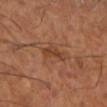• biopsy status · imaged on a skin check; not biopsied
• site · the leg
• image · 15 mm crop, total-body photography
• tile lighting · cross-polarized illumination
• size · ≈3.5 mm
• patient · male, aged approximately 65